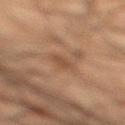A male subject, about 50 years old. A close-up tile cropped from a whole-body skin photograph, about 15 mm across. An algorithmic analysis of the crop reported a lesion area of about 3.5 mm² and two-axis asymmetry of about 0.25. And it measured a mean CIELAB color near L≈36 a*≈16 b*≈25 and a lesion-to-skin contrast of about 6 (normalized; higher = more distinct). From the leg. This is a cross-polarized tile. The lesion's longest dimension is about 2.5 mm.